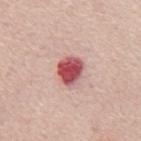{
  "biopsy_status": "not biopsied; imaged during a skin examination",
  "automated_metrics": {
    "eccentricity": 0.6,
    "shape_asymmetry": 0.2,
    "cielab_L": 53,
    "cielab_a": 34,
    "cielab_b": 22,
    "vs_skin_darker_L": 19.0,
    "vs_skin_contrast_norm": 12.0,
    "border_irregularity_0_10": 1.5,
    "peripheral_color_asymmetry": 1.5
  },
  "site": "mid back",
  "patient": {
    "sex": "male",
    "age_approx": 65
  },
  "image": {
    "source": "total-body photography crop",
    "field_of_view_mm": 15
  }
}